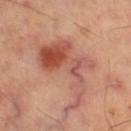| field | value |
|---|---|
| size | ≈9 mm |
| image source | 15 mm crop, total-body photography |
| site | the leg |